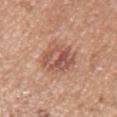Clinical impression: The lesion was photographed on a routine skin check and not biopsied; there is no pathology result. Context: The lesion-visualizer software estimated an area of roughly 13 mm², an outline eccentricity of about 0.8 (0 = round, 1 = elongated), and a shape-asymmetry score of about 0.25 (0 = symmetric). The software also gave a peripheral color-asymmetry measure near 3. The analysis additionally found lesion-presence confidence of about 100/100. A close-up tile cropped from a whole-body skin photograph, about 15 mm across. The subject is a female roughly 50 years of age. Captured under white-light illumination. About 5.5 mm across. The lesion is on the left upper arm.